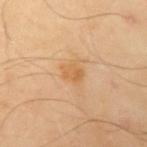notes — catalogued during a skin exam; not biopsied
illumination — cross-polarized
anatomic site — the left upper arm
diameter — about 2.5 mm
patient — male, aged 53 to 57
image source — ~15 mm crop, total-body skin-cancer survey
TBP lesion metrics — a lesion area of about 4 mm², a shape eccentricity near 0.6, and a shape-asymmetry score of about 0.25 (0 = symmetric); a classifier nevus-likeness of about 15/100